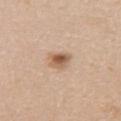- notes: no biopsy performed (imaged during a skin exam)
- subject: male, approximately 40 years of age
- illumination: white-light
- location: the left upper arm
- lesion diameter: about 2.5 mm
- imaging modality: ~15 mm tile from a whole-body skin photo